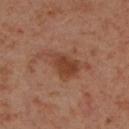  biopsy_status: not biopsied; imaged during a skin examination
  image:
    source: total-body photography crop
    field_of_view_mm: 15
  site: leg
  lesion_size:
    long_diameter_mm_approx: 6.5
  patient:
    sex: male
    age_approx: 55